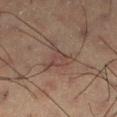<case>
<biopsy_status>not biopsied; imaged during a skin examination</biopsy_status>
<image>
  <source>total-body photography crop</source>
  <field_of_view_mm>15</field_of_view_mm>
</image>
<automated_metrics>
  <border_irregularity_0_10>7.5</border_irregularity_0_10>
  <color_variation_0_10>2.0</color_variation_0_10>
  <peripheral_color_asymmetry>0.5</peripheral_color_asymmetry>
</automated_metrics>
<lighting>cross-polarized</lighting>
<patient>
  <sex>male</sex>
  <age_approx>60</age_approx>
</patient>
<lesion_size>
  <long_diameter_mm_approx>4.0</long_diameter_mm_approx>
</lesion_size>
</case>